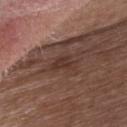Q: Is there a histopathology result?
A: total-body-photography surveillance lesion; no biopsy
Q: Who is the patient?
A: male, in their mid- to late 70s
Q: What kind of image is this?
A: 15 mm crop, total-body photography
Q: Lesion location?
A: the chest
Q: What is the lesion's diameter?
A: ≈3.5 mm
Q: What did automated image analysis measure?
A: roughly 7 lightness units darker than nearby skin; a nevus-likeness score of about 5/100 and a detector confidence of about 95 out of 100 that the crop contains a lesion